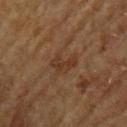Captured during whole-body skin photography for melanoma surveillance; the lesion was not biopsied. A male patient, approximately 65 years of age. The lesion-visualizer software estimated a border-irregularity rating of about 5.5/10, internal color variation of about 2 on a 0–10 scale, and a peripheral color-asymmetry measure near 0.5. And it measured a classifier nevus-likeness of about 0/100 and a lesion-detection confidence of about 100/100. Approximately 3.5 mm at its widest. From the upper back. A roughly 15 mm field-of-view crop from a total-body skin photograph.